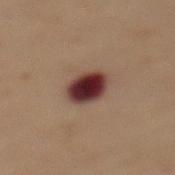Context: Automated tile analysis of the lesion measured a footprint of about 9.5 mm², a shape eccentricity near 0.75, and a shape-asymmetry score of about 0.15 (0 = symmetric). It also reported a nevus-likeness score of about 10/100 and lesion-presence confidence of about 100/100. Captured under cross-polarized illumination. A female subject, in their 50s. A lesion tile, about 15 mm wide, cut from a 3D total-body photograph. From the mid back.